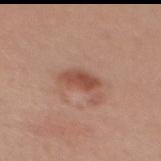Imaged during a routine full-body skin examination; the lesion was not biopsied and no histopathology is available.
The lesion's longest dimension is about 4 mm.
Cropped from a whole-body photographic skin survey; the tile spans about 15 mm.
On the mid back.
The lesion-visualizer software estimated an area of roughly 6 mm², a shape eccentricity near 0.85, and a shape-asymmetry score of about 0.2 (0 = symmetric). The software also gave roughly 11 lightness units darker than nearby skin and a lesion-to-skin contrast of about 8 (normalized; higher = more distinct).
A female subject aged approximately 25.
Imaged with white-light lighting.